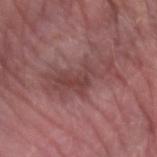workup = catalogued during a skin exam; not biopsied
acquisition = ~15 mm crop, total-body skin-cancer survey
anatomic site = the left forearm
automated lesion analysis = a classifier nevus-likeness of about 0/100 and lesion-presence confidence of about 80/100
subject = male, roughly 80 years of age